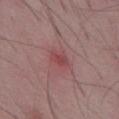This lesion was catalogued during total-body skin photography and was not selected for biopsy. The patient is a male about 50 years old. A region of skin cropped from a whole-body photographic capture, roughly 15 mm wide. On the front of the torso.